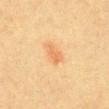image source — ~15 mm tile from a whole-body skin photo | automated metrics — a lesion area of about 3.5 mm², a shape eccentricity near 0.9, and two-axis asymmetry of about 0.3; a lesion color around L≈61 a*≈22 b*≈39 in CIELAB, roughly 8 lightness units darker than nearby skin, and a lesion-to-skin contrast of about 5.5 (normalized; higher = more distinct) | body site — the back | lesion size — ~3 mm (longest diameter) | subject — female, approximately 55 years of age | tile lighting — cross-polarized illumination.